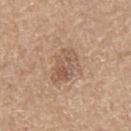Impression:
Captured during whole-body skin photography for melanoma surveillance; the lesion was not biopsied.
Clinical summary:
From the leg. The lesion's longest dimension is about 4.5 mm. This is a white-light tile. A close-up tile cropped from a whole-body skin photograph, about 15 mm across. Automated image analysis of the tile measured a border-irregularity rating of about 3.5/10 and a color-variation rating of about 5/10. The patient is a female aged around 70.